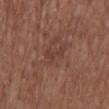Recorded during total-body skin imaging; not selected for excision or biopsy.
From the head or neck.
Cropped from a whole-body photographic skin survey; the tile spans about 15 mm.
The lesion's longest dimension is about 2.5 mm.
The subject is a female approximately 75 years of age.
The tile uses white-light illumination.